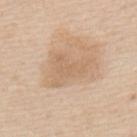Image and clinical context:
Approximately 6 mm at its widest. Automated image analysis of the tile measured an area of roughly 13 mm², an eccentricity of roughly 0.8, and a symmetry-axis asymmetry near 0.5. The analysis additionally found border irregularity of about 5.5 on a 0–10 scale, a within-lesion color-variation index near 2/10, and a peripheral color-asymmetry measure near 0.5. And it measured a nevus-likeness score of about 0/100 and a lesion-detection confidence of about 100/100. A 15 mm close-up tile from a total-body photography series done for melanoma screening. A male patient in their mid- to late 60s. The lesion is located on the upper back.